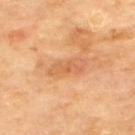The subject is a male roughly 70 years of age. About 4.5 mm across. Cropped from a total-body skin-imaging series; the visible field is about 15 mm. Automated tile analysis of the lesion measured a symmetry-axis asymmetry near 0.25. And it measured an average lesion color of about L≈62 a*≈25 b*≈40 (CIELAB) and a normalized border contrast of about 5. The software also gave a border-irregularity index near 3/10 and a color-variation rating of about 4/10. The lesion is located on the upper back.